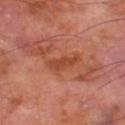Clinical impression: This lesion was catalogued during total-body skin photography and was not selected for biopsy.